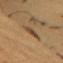Q: Is there a histopathology result?
A: total-body-photography surveillance lesion; no biopsy
Q: Automated lesion metrics?
A: a lesion area of about 3.5 mm², a shape eccentricity near 0.95, and two-axis asymmetry of about 0.25; a mean CIELAB color near L≈46 a*≈16 b*≈33, roughly 10 lightness units darker than nearby skin, and a normalized border contrast of about 8; a border-irregularity index near 3.5/10, a within-lesion color-variation index near 2/10, and radial color variation of about 0.5; lesion-presence confidence of about 60/100
Q: Lesion location?
A: the right upper arm
Q: How was the tile lit?
A: cross-polarized illumination
Q: Patient demographics?
A: female, aged around 55
Q: What is the lesion's diameter?
A: about 3.5 mm
Q: How was this image acquired?
A: 15 mm crop, total-body photography